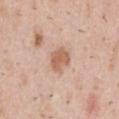Findings:
– notes · imaged on a skin check; not biopsied
– lesion size · ≈3 mm
– image · total-body-photography crop, ~15 mm field of view
– subject · male, aged around 40
– anatomic site · the abdomen
– automated metrics · a lesion area of about 6 mm², an outline eccentricity of about 0.5 (0 = round, 1 = elongated), and a shape-asymmetry score of about 0.2 (0 = symmetric); an average lesion color of about L≈61 a*≈21 b*≈31 (CIELAB), a lesion–skin lightness drop of about 11, and a normalized border contrast of about 7.5; border irregularity of about 2 on a 0–10 scale, a within-lesion color-variation index near 2/10, and peripheral color asymmetry of about 0.5